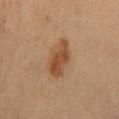Notes:
* notes: total-body-photography surveillance lesion; no biopsy
* patient: female, aged approximately 60
* image source: total-body-photography crop, ~15 mm field of view
* location: the mid back